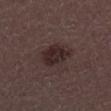Recorded during total-body skin imaging; not selected for excision or biopsy. This is a white-light tile. The recorded lesion diameter is about 4 mm. A male patient aged 53 to 57. The lesion is on the leg. A 15 mm crop from a total-body photograph taken for skin-cancer surveillance.